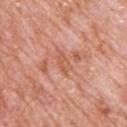- notes · no biopsy performed (imaged during a skin exam)
- size · about 2.5 mm
- automated metrics · an area of roughly 3.5 mm², an outline eccentricity of about 0.85 (0 = round, 1 = elongated), and a symmetry-axis asymmetry near 0.25; a lesion color around L≈58 a*≈27 b*≈34 in CIELAB and a lesion–skin lightness drop of about 7; internal color variation of about 3 on a 0–10 scale and peripheral color asymmetry of about 1; a nevus-likeness score of about 0/100
- acquisition · ~15 mm crop, total-body skin-cancer survey
- patient · male, aged 78–82
- site · the upper back
- lighting · white-light